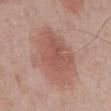No biopsy was performed on this lesion — it was imaged during a full skin examination and was not determined to be concerning. On the chest. The subject is a male aged 68 to 72. This is a white-light tile. Cropped from a total-body skin-imaging series; the visible field is about 15 mm. The recorded lesion diameter is about 7.5 mm.